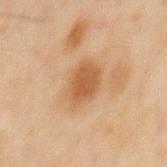A male subject about 45 years old.
The lesion is located on the mid back.
A region of skin cropped from a whole-body photographic capture, roughly 15 mm wide.